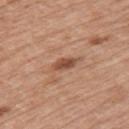Part of a total-body skin-imaging series; this lesion was reviewed on a skin check and was not flagged for biopsy. Approximately 3 mm at its widest. A region of skin cropped from a whole-body photographic capture, roughly 15 mm wide. Imaged with white-light lighting. The lesion is on the left upper arm. The subject is a female aged 38 to 42.